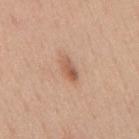Impression:
This lesion was catalogued during total-body skin photography and was not selected for biopsy.
Clinical summary:
Captured under white-light illumination. A region of skin cropped from a whole-body photographic capture, roughly 15 mm wide. The lesion is located on the mid back. A male subject aged 28–32. The lesion's longest dimension is about 3.5 mm.